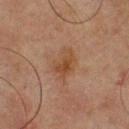Q: Was a biopsy performed?
A: total-body-photography surveillance lesion; no biopsy
Q: What lighting was used for the tile?
A: cross-polarized
Q: Who is the patient?
A: male, approximately 65 years of age
Q: How was this image acquired?
A: total-body-photography crop, ~15 mm field of view
Q: What is the anatomic site?
A: the upper back